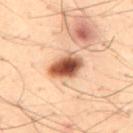{"biopsy_status": "not biopsied; imaged during a skin examination", "site": "back", "lighting": "cross-polarized", "patient": {"sex": "male", "age_approx": 30}, "lesion_size": {"long_diameter_mm_approx": 4.5}, "image": {"source": "total-body photography crop", "field_of_view_mm": 15}}